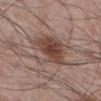The lesion was photographed on a routine skin check and not biopsied; there is no pathology result. Automated tile analysis of the lesion measured a lesion area of about 19 mm², an eccentricity of roughly 0.75, and a symmetry-axis asymmetry near 0.4. The software also gave an average lesion color of about L≈46 a*≈18 b*≈23 (CIELAB), about 11 CIELAB-L* units darker than the surrounding skin, and a lesion-to-skin contrast of about 8 (normalized; higher = more distinct). The software also gave border irregularity of about 5.5 on a 0–10 scale and a within-lesion color-variation index near 4.5/10. And it measured a nevus-likeness score of about 95/100 and a lesion-detection confidence of about 100/100. A close-up tile cropped from a whole-body skin photograph, about 15 mm across. Longest diameter approximately 6.5 mm. The lesion is located on the right lower leg. This is a white-light tile. A male subject, about 60 years old.